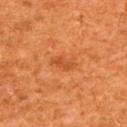Background:
The lesion is on the upper back. A 15 mm close-up extracted from a 3D total-body photography capture. The patient is a male aged around 65. Captured under cross-polarized illumination.